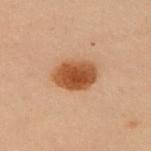Part of a total-body skin-imaging series; this lesion was reviewed on a skin check and was not flagged for biopsy. A female subject, in their 50s. This is a cross-polarized tile. This image is a 15 mm lesion crop taken from a total-body photograph. About 4.5 mm across. From the left upper arm. The lesion-visualizer software estimated a lesion–skin lightness drop of about 14 and a lesion-to-skin contrast of about 11 (normalized; higher = more distinct).